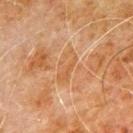Findings:
* notes · total-body-photography surveillance lesion; no biopsy
* patient · male, aged 78 to 82
* TBP lesion metrics · a lesion area of about 6.5 mm², a shape eccentricity near 0.9, and two-axis asymmetry of about 0.15; a normalized lesion–skin contrast near 4.5
* image · total-body-photography crop, ~15 mm field of view
* lighting · cross-polarized
* body site · the left upper arm
* lesion diameter · about 4.5 mm